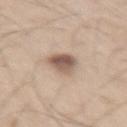Case summary:
• biopsy status — catalogued during a skin exam; not biopsied
• patient — male, about 60 years old
• acquisition — 15 mm crop, total-body photography
• lesion size — about 3.5 mm
• TBP lesion metrics — a nevus-likeness score of about 80/100 and a lesion-detection confidence of about 100/100
• anatomic site — the right thigh
• tile lighting — white-light illumination